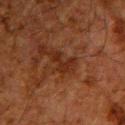Recorded during total-body skin imaging; not selected for excision or biopsy. The total-body-photography lesion software estimated a shape eccentricity near 0.85 and a symmetry-axis asymmetry near 0.65. Imaged with cross-polarized lighting. A region of skin cropped from a whole-body photographic capture, roughly 15 mm wide. The lesion is located on the upper back. The subject is a male about 60 years old.